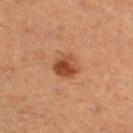Assessment:
The lesion was tiled from a total-body skin photograph and was not biopsied.
Background:
Longest diameter approximately 3 mm. A lesion tile, about 15 mm wide, cut from a 3D total-body photograph. The subject is a female roughly 55 years of age. The lesion is on the right thigh.